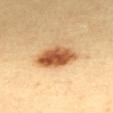Clinical impression:
Imaged during a routine full-body skin examination; the lesion was not biopsied and no histopathology is available.
Image and clinical context:
The patient is a female aged approximately 40. This image is a 15 mm lesion crop taken from a total-body photograph. Located on the mid back. The total-body-photography lesion software estimated a lesion area of about 12 mm², an eccentricity of roughly 0.9, and two-axis asymmetry of about 0.2.